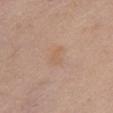workup: imaged on a skin check; not biopsied
site: the chest
patient: female, approximately 65 years of age
automated metrics: a lesion area of about 3 mm², an eccentricity of roughly 0.85, and a shape-asymmetry score of about 0.4 (0 = symmetric); a border-irregularity index near 4/10 and a within-lesion color-variation index near 0.5/10; an automated nevus-likeness rating near 0 out of 100 and lesion-presence confidence of about 100/100
imaging modality: total-body-photography crop, ~15 mm field of view
lesion diameter: ~2.5 mm (longest diameter)
tile lighting: white-light illumination